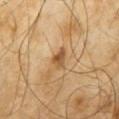follow-up=total-body-photography surveillance lesion; no biopsy
location=the mid back
image-analysis metrics=an area of roughly 4 mm², a shape eccentricity near 0.65, and two-axis asymmetry of about 0.35; an average lesion color of about L≈50 a*≈19 b*≈37 (CIELAB), a lesion–skin lightness drop of about 11, and a normalized lesion–skin contrast near 8; peripheral color asymmetry of about 1.5
acquisition=~15 mm tile from a whole-body skin photo
patient=male, in their mid- to late 60s